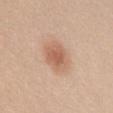Q: Was this lesion biopsied?
A: no biopsy performed (imaged during a skin exam)
Q: Automated lesion metrics?
A: a mean CIELAB color near L≈61 a*≈21 b*≈31, roughly 10 lightness units darker than nearby skin, and a normalized border contrast of about 6.5
Q: Lesion location?
A: the mid back
Q: What kind of image is this?
A: total-body-photography crop, ~15 mm field of view
Q: Lesion size?
A: ~4 mm (longest diameter)
Q: Illumination type?
A: white-light
Q: What are the patient's age and sex?
A: female, aged 28–32